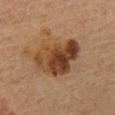Recorded during total-body skin imaging; not selected for excision or biopsy. On the mid back. The tile uses cross-polarized illumination. A male subject, aged 58–62. Approximately 6 mm at its widest. A region of skin cropped from a whole-body photographic capture, roughly 15 mm wide.